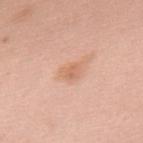{"biopsy_status": "not biopsied; imaged during a skin examination", "lesion_size": {"long_diameter_mm_approx": 2.5}, "lighting": "white-light", "image": {"source": "total-body photography crop", "field_of_view_mm": 15}, "site": "upper back", "patient": {"sex": "female", "age_approx": 65}}